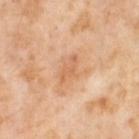Findings:
– workup · catalogued during a skin exam; not biopsied
– tile lighting · cross-polarized
– automated metrics · border irregularity of about 4 on a 0–10 scale and peripheral color asymmetry of about 0.5
– imaging modality · ~15 mm crop, total-body skin-cancer survey
– patient · female, in their mid-50s
– body site · the right thigh
– lesion size · about 3.5 mm